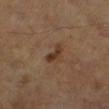Recorded during total-body skin imaging; not selected for excision or biopsy. The subject is a male aged 63 to 67. A 15 mm close-up tile from a total-body photography series done for melanoma screening. From the left lower leg.